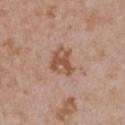Notes:
– biopsy status · imaged on a skin check; not biopsied
– patient · female, aged around 30
– tile lighting · white-light
– acquisition · ~15 mm crop, total-body skin-cancer survey
– lesion size · about 4 mm
– location · the chest